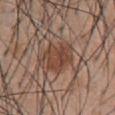notes: imaged on a skin check; not biopsied
tile lighting: white-light
site: the abdomen
lesion diameter: about 4 mm
subject: male, aged around 55
automated metrics: border irregularity of about 3.5 on a 0–10 scale, a color-variation rating of about 4.5/10, and radial color variation of about 1.5
acquisition: total-body-photography crop, ~15 mm field of view